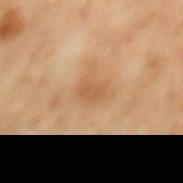workup = imaged on a skin check; not biopsied | automated metrics = an area of roughly 4 mm² and a symmetry-axis asymmetry near 0.2; an average lesion color of about L≈53 a*≈20 b*≈35 (CIELAB) and a normalized border contrast of about 5.5 | image source = ~15 mm tile from a whole-body skin photo | lesion size = about 2.5 mm | site = the back | subject = male, aged 58 to 62.